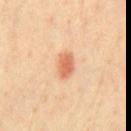workup = total-body-photography surveillance lesion; no biopsy
location = the chest
imaging modality = ~15 mm crop, total-body skin-cancer survey
patient = male, roughly 40 years of age
lesion size = ~3.5 mm (longest diameter)